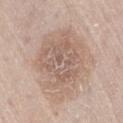Recorded during total-body skin imaging; not selected for excision or biopsy.
A male patient, aged 63 to 67.
Imaged with white-light lighting.
Cropped from a total-body skin-imaging series; the visible field is about 15 mm.
The lesion is on the right thigh.
The recorded lesion diameter is about 8 mm.